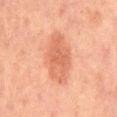- subject: male, approximately 65 years of age
- anatomic site: the abdomen
- imaging modality: ~15 mm crop, total-body skin-cancer survey
- size: ≈7.5 mm